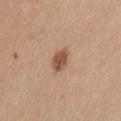workup — total-body-photography surveillance lesion; no biopsy
patient — female, approximately 40 years of age
automated lesion analysis — a footprint of about 5 mm² and an eccentricity of roughly 0.7; a lesion–skin lightness drop of about 12 and a normalized border contrast of about 8.5; border irregularity of about 1.5 on a 0–10 scale and a within-lesion color-variation index near 2.5/10; a nevus-likeness score of about 95/100
diameter — ~3 mm (longest diameter)
image source — ~15 mm tile from a whole-body skin photo
illumination — white-light
body site — the back